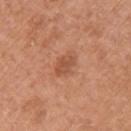Captured during whole-body skin photography for melanoma surveillance; the lesion was not biopsied. A 15 mm crop from a total-body photograph taken for skin-cancer surveillance. Located on the upper back. Imaged with white-light lighting. A female patient, aged approximately 65.